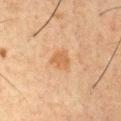Case summary:
- notes — imaged on a skin check; not biopsied
- body site — the front of the torso
- subject — male, aged approximately 50
- tile lighting — cross-polarized
- automated lesion analysis — an eccentricity of roughly 0.35; a border-irregularity rating of about 2/10 and a within-lesion color-variation index near 2/10; a nevus-likeness score of about 55/100 and a detector confidence of about 100 out of 100 that the crop contains a lesion
- acquisition — 15 mm crop, total-body photography